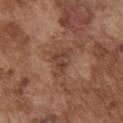Q: Was this lesion biopsied?
A: no biopsy performed (imaged during a skin exam)
Q: Illumination type?
A: white-light illumination
Q: What are the patient's age and sex?
A: male, aged 73–77
Q: Lesion location?
A: the chest
Q: What kind of image is this?
A: ~15 mm tile from a whole-body skin photo
Q: Lesion size?
A: ~4 mm (longest diameter)